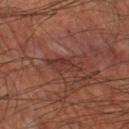{"lighting": "cross-polarized", "site": "leg", "image": {"source": "total-body photography crop", "field_of_view_mm": 15}, "lesion_size": {"long_diameter_mm_approx": 4.5}, "patient": {"sex": "male", "age_approx": 60}}